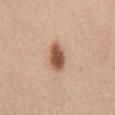No biopsy was performed on this lesion — it was imaged during a full skin examination and was not determined to be concerning. The total-body-photography lesion software estimated a border-irregularity index near 1.5/10, a within-lesion color-variation index near 4.5/10, and a peripheral color-asymmetry measure near 1.5. The software also gave lesion-presence confidence of about 100/100. A male patient, aged approximately 60. Captured under white-light illumination. Measured at roughly 4 mm in maximum diameter. The lesion is located on the mid back. A region of skin cropped from a whole-body photographic capture, roughly 15 mm wide.